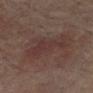Q: Was a biopsy performed?
A: imaged on a skin check; not biopsied
Q: Lesion location?
A: the right lower leg
Q: Who is the patient?
A: female, in their 80s
Q: How was this image acquired?
A: ~15 mm tile from a whole-body skin photo